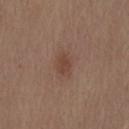{
  "biopsy_status": "not biopsied; imaged during a skin examination",
  "lighting": "white-light",
  "image": {
    "source": "total-body photography crop",
    "field_of_view_mm": 15
  },
  "patient": {
    "sex": "male",
    "age_approx": 70
  },
  "site": "mid back",
  "automated_metrics": {
    "area_mm2_approx": 4.0,
    "eccentricity": 0.7,
    "shape_asymmetry": 0.25,
    "color_variation_0_10": 1.0,
    "peripheral_color_asymmetry": 0.5,
    "lesion_detection_confidence_0_100": 100
  }
}